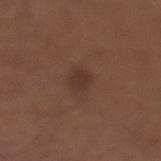This image is a 15 mm lesion crop taken from a total-body photograph.
From the left lower leg.
A female patient, approximately 55 years of age.
The tile uses white-light illumination.
Measured at roughly 2.5 mm in maximum diameter.